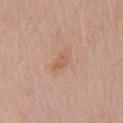Q: Is there a histopathology result?
A: no biopsy performed (imaged during a skin exam)
Q: How was the tile lit?
A: white-light
Q: What is the lesion's diameter?
A: ≈3 mm
Q: What kind of image is this?
A: total-body-photography crop, ~15 mm field of view
Q: What did automated image analysis measure?
A: an average lesion color of about L≈59 a*≈21 b*≈31 (CIELAB), roughly 6 lightness units darker than nearby skin, and a normalized border contrast of about 5; border irregularity of about 2 on a 0–10 scale, a color-variation rating of about 3/10, and peripheral color asymmetry of about 1; an automated nevus-likeness rating near 0 out of 100 and lesion-presence confidence of about 100/100
Q: Where on the body is the lesion?
A: the front of the torso
Q: What are the patient's age and sex?
A: female, in their mid- to late 60s